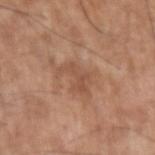Part of a total-body skin-imaging series; this lesion was reviewed on a skin check and was not flagged for biopsy. The lesion-visualizer software estimated a lesion color around L≈51 a*≈22 b*≈31 in CIELAB, a lesion–skin lightness drop of about 7, and a normalized lesion–skin contrast near 5.5. The software also gave border irregularity of about 7 on a 0–10 scale and internal color variation of about 1.5 on a 0–10 scale. The subject is a male in their mid- to late 70s. Captured under white-light illumination. Measured at roughly 4 mm in maximum diameter. Cropped from a whole-body photographic skin survey; the tile spans about 15 mm. The lesion is located on the left upper arm.